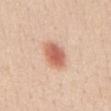Impression:
Recorded during total-body skin imaging; not selected for excision or biopsy.
Background:
A 15 mm close-up tile from a total-body photography series done for melanoma screening. From the front of the torso. A female patient, in their mid- to late 40s.